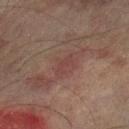Q: What kind of image is this?
A: 15 mm crop, total-body photography
Q: What are the patient's age and sex?
A: male, in their mid- to late 70s
Q: Where on the body is the lesion?
A: the right lower leg
Q: Illumination type?
A: cross-polarized illumination
Q: What is the lesion's diameter?
A: ~2.5 mm (longest diameter)